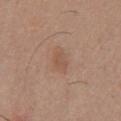workup: total-body-photography surveillance lesion; no biopsy
subject: male, aged approximately 55
TBP lesion metrics: a lesion area of about 5.5 mm², a shape eccentricity near 0.8, and a shape-asymmetry score of about 0.25 (0 = symmetric); a lesion-to-skin contrast of about 4.5 (normalized; higher = more distinct); border irregularity of about 2.5 on a 0–10 scale, a within-lesion color-variation index near 1.5/10, and radial color variation of about 0.5; a classifier nevus-likeness of about 5/100
tile lighting: white-light illumination
site: the chest
diameter: ~3.5 mm (longest diameter)
image source: total-body-photography crop, ~15 mm field of view